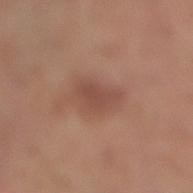Part of a total-body skin-imaging series; this lesion was reviewed on a skin check and was not flagged for biopsy. On the right lower leg. Cropped from a whole-body photographic skin survey; the tile spans about 15 mm. A female patient in their mid-60s. The tile uses white-light illumination.